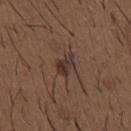notes: no biopsy performed (imaged during a skin exam) | lesion size: ≈3.5 mm | image source: total-body-photography crop, ~15 mm field of view | illumination: white-light illumination | TBP lesion metrics: about 8 CIELAB-L* units darker than the surrounding skin and a normalized lesion–skin contrast near 8.5; a border-irregularity index near 3.5/10 and internal color variation of about 4.5 on a 0–10 scale; a lesion-detection confidence of about 100/100 | subject: male, aged 48–52 | location: the chest.